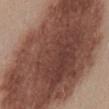Q: Was a biopsy performed?
A: imaged on a skin check; not biopsied
Q: What did automated image analysis measure?
A: a lesion area of about 185 mm², an eccentricity of roughly 0.85, and a symmetry-axis asymmetry near 0.15; roughly 18 lightness units darker than nearby skin and a lesion-to-skin contrast of about 12.5 (normalized; higher = more distinct); a within-lesion color-variation index near 7/10 and a peripheral color-asymmetry measure near 2.5; a nevus-likeness score of about 80/100 and a lesion-detection confidence of about 100/100
Q: How was this image acquired?
A: ~15 mm tile from a whole-body skin photo
Q: How was the tile lit?
A: white-light illumination
Q: What is the anatomic site?
A: the front of the torso
Q: What is the lesion's diameter?
A: about 20.5 mm
Q: Patient demographics?
A: female, in their mid-40s